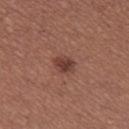{
  "biopsy_status": "not biopsied; imaged during a skin examination",
  "patient": {
    "sex": "female",
    "age_approx": 25
  },
  "image": {
    "source": "total-body photography crop",
    "field_of_view_mm": 15
  },
  "site": "left thigh",
  "lighting": "white-light"
}